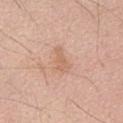* follow-up — no biopsy performed (imaged during a skin exam)
* lesion size — ≈3 mm
* automated metrics — a nevus-likeness score of about 0/100 and a lesion-detection confidence of about 100/100
* patient — male, aged around 70
* anatomic site — the abdomen
* image source — ~15 mm tile from a whole-body skin photo